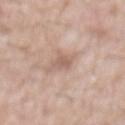A male patient, in their 60s.
An algorithmic analysis of the crop reported a mean CIELAB color near L≈60 a*≈18 b*≈26, about 9 CIELAB-L* units darker than the surrounding skin, and a normalized border contrast of about 5.5. It also reported a nevus-likeness score of about 0/100.
On the chest.
The tile uses white-light illumination.
A 15 mm close-up extracted from a 3D total-body photography capture.
Longest diameter approximately 2.5 mm.